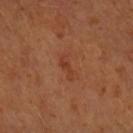The lesion was photographed on a routine skin check and not biopsied; there is no pathology result.
Located on the right forearm.
The patient is female.
The lesion-visualizer software estimated a shape eccentricity near 0.95 and a shape-asymmetry score of about 0.35 (0 = symmetric). And it measured border irregularity of about 4.5 on a 0–10 scale, a within-lesion color-variation index near 0/10, and a peripheral color-asymmetry measure near 0. The software also gave an automated nevus-likeness rating near 0 out of 100 and a lesion-detection confidence of about 100/100.
Approximately 3 mm at its widest.
Captured under cross-polarized illumination.
A 15 mm close-up extracted from a 3D total-body photography capture.